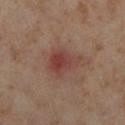Imaged during a routine full-body skin examination; the lesion was not biopsied and no histopathology is available. A female subject about 55 years old. The lesion's longest dimension is about 4.5 mm. A lesion tile, about 15 mm wide, cut from a 3D total-body photograph. The lesion is on the right lower leg. Captured under cross-polarized illumination. The lesion-visualizer software estimated a footprint of about 10 mm² and an outline eccentricity of about 0.7 (0 = round, 1 = elongated). It also reported border irregularity of about 3.5 on a 0–10 scale, a within-lesion color-variation index near 5.5/10, and radial color variation of about 2.